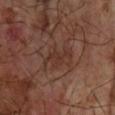The lesion was photographed on a routine skin check and not biopsied; there is no pathology result.
The lesion's longest dimension is about 3.5 mm.
The lesion is located on the right forearm.
A male subject aged 63 to 67.
A 15 mm crop from a total-body photograph taken for skin-cancer surveillance.
Automated image analysis of the tile measured a lesion area of about 4.5 mm² and a shape-asymmetry score of about 0.5 (0 = symmetric). It also reported a classifier nevus-likeness of about 0/100 and a lesion-detection confidence of about 95/100.